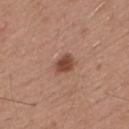patient=male, aged around 55
lesion size=~2.5 mm (longest diameter)
body site=the mid back
acquisition=15 mm crop, total-body photography
tile lighting=white-light